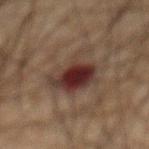biopsy status: imaged on a skin check; not biopsied | patient: male, roughly 65 years of age | lesion diameter: ~5 mm (longest diameter) | automated metrics: a shape eccentricity near 0.25 and a symmetry-axis asymmetry near 0.3; border irregularity of about 4.5 on a 0–10 scale, internal color variation of about 7.5 on a 0–10 scale, and radial color variation of about 2 | location: the abdomen | lighting: cross-polarized illumination | imaging modality: ~15 mm tile from a whole-body skin photo.